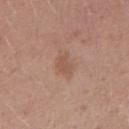{
  "biopsy_status": "not biopsied; imaged during a skin examination",
  "site": "right upper arm",
  "image": {
    "source": "total-body photography crop",
    "field_of_view_mm": 15
  },
  "patient": {
    "sex": "male",
    "age_approx": 25
  }
}